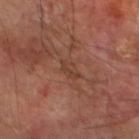notes: no biopsy performed (imaged during a skin exam); image source: ~15 mm tile from a whole-body skin photo; diameter: ~3 mm (longest diameter); anatomic site: the leg; tile lighting: cross-polarized; subject: male, aged around 70.